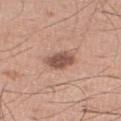{
  "biopsy_status": "not biopsied; imaged during a skin examination",
  "image": {
    "source": "total-body photography crop",
    "field_of_view_mm": 15
  },
  "patient": {
    "sex": "male",
    "age_approx": 20
  },
  "lighting": "white-light",
  "site": "right lower leg",
  "lesion_size": {
    "long_diameter_mm_approx": 3.5
  }
}